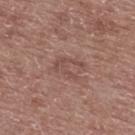  biopsy_status: not biopsied; imaged during a skin examination
  lighting: white-light
  lesion_size:
    long_diameter_mm_approx: 3.5
  image:
    source: total-body photography crop
    field_of_view_mm: 15
  patient:
    sex: male
    age_approx: 60
  site: upper back
  automated_metrics:
    area_mm2_approx: 5.0
    eccentricity: 0.7
    color_variation_0_10: 1.0
    peripheral_color_asymmetry: 0.0